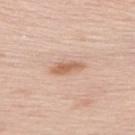Findings:
- biopsy status: imaged on a skin check; not biopsied
- patient: female, roughly 65 years of age
- imaging modality: total-body-photography crop, ~15 mm field of view
- site: the upper back